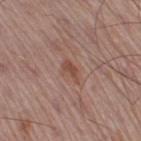follow-up: no biopsy performed (imaged during a skin exam); size: ≈2.5 mm; image: ~15 mm tile from a whole-body skin photo; patient: male, roughly 55 years of age; tile lighting: white-light illumination; location: the leg.